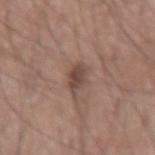Notes:
• follow-up: imaged on a skin check; not biopsied
• subject: male, aged approximately 55
• acquisition: ~15 mm crop, total-body skin-cancer survey
• diameter: ~2.5 mm (longest diameter)
• tile lighting: white-light